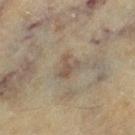  biopsy_status: not biopsied; imaged during a skin examination
  patient:
    sex: female
    age_approx: 55
  lesion_size:
    long_diameter_mm_approx: 3.0
  site: left thigh
  lighting: cross-polarized
  image:
    source: total-body photography crop
    field_of_view_mm: 15